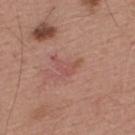- workup: no biopsy performed (imaged during a skin exam)